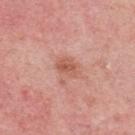Captured during whole-body skin photography for melanoma surveillance; the lesion was not biopsied. The recorded lesion diameter is about 2.5 mm. Captured under white-light illumination. The subject is a male about 65 years old. A 15 mm close-up tile from a total-body photography series done for melanoma screening. From the upper back.